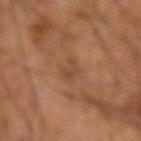- biopsy status — imaged on a skin check; not biopsied
- TBP lesion metrics — a footprint of about 4.5 mm², an outline eccentricity of about 0.7 (0 = round, 1 = elongated), and two-axis asymmetry of about 0.25; a lesion color around L≈45 a*≈20 b*≈31 in CIELAB and a normalized border contrast of about 5
- body site — the right forearm
- imaging modality — 15 mm crop, total-body photography
- subject — male, aged approximately 45